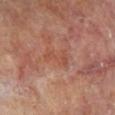Q: Was this lesion biopsied?
A: total-body-photography surveillance lesion; no biopsy
Q: How large is the lesion?
A: ~3.5 mm (longest diameter)
Q: Lesion location?
A: the left lower leg
Q: What kind of image is this?
A: ~15 mm tile from a whole-body skin photo
Q: What are the patient's age and sex?
A: female, in their mid- to late 70s
Q: How was the tile lit?
A: cross-polarized illumination